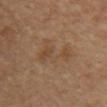notes=catalogued during a skin exam; not biopsied
body site=the abdomen
image-analysis metrics=a lesion area of about 10 mm², a shape eccentricity near 0.8, and two-axis asymmetry of about 0.65; a border-irregularity index near 9/10, internal color variation of about 3 on a 0–10 scale, and peripheral color asymmetry of about 1
lesion diameter=about 5.5 mm
subject=male, aged around 65
lighting=cross-polarized illumination
image=15 mm crop, total-body photography